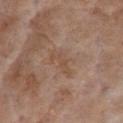biopsy status = imaged on a skin check; not biopsied
anatomic site = the chest
automated metrics = a border-irregularity rating of about 5.5/10, a color-variation rating of about 0/10, and peripheral color asymmetry of about 0; a classifier nevus-likeness of about 0/100 and lesion-presence confidence of about 100/100
diameter = ~3.5 mm (longest diameter)
illumination = white-light
subject = female, aged 83 or older
image = total-body-photography crop, ~15 mm field of view